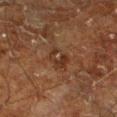biopsy status=no biopsy performed (imaged during a skin exam)
automated lesion analysis=a lesion area of about 5 mm²; a border-irregularity index near 6/10, a color-variation rating of about 2/10, and a peripheral color-asymmetry measure near 0.5
lesion size=~3 mm (longest diameter)
patient=male, about 60 years old
tile lighting=cross-polarized
anatomic site=the left lower leg
image source=total-body-photography crop, ~15 mm field of view